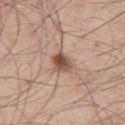Assessment:
No biopsy was performed on this lesion — it was imaged during a full skin examination and was not determined to be concerning.
Context:
About 3 mm across. The tile uses white-light illumination. This image is a 15 mm lesion crop taken from a total-body photograph. The lesion is located on the left thigh. A male subject, roughly 45 years of age.